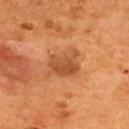Clinical impression: No biopsy was performed on this lesion — it was imaged during a full skin examination and was not determined to be concerning. Acquisition and patient details: Captured under cross-polarized illumination. A male patient aged 53–57. A 15 mm crop from a total-body photograph taken for skin-cancer surveillance. Located on the upper back. Automated image analysis of the tile measured an area of roughly 8.5 mm² and two-axis asymmetry of about 0.35. And it measured roughly 10 lightness units darker than nearby skin and a normalized border contrast of about 7.